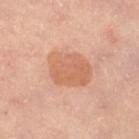{
  "biopsy_status": "not biopsied; imaged during a skin examination",
  "image": {
    "source": "total-body photography crop",
    "field_of_view_mm": 15
  },
  "patient": {
    "sex": "female",
    "age_approx": 45
  },
  "site": "leg",
  "lesion_size": {
    "long_diameter_mm_approx": 5.0
  }
}